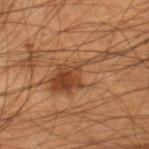| feature | finding |
|---|---|
| notes | catalogued during a skin exam; not biopsied |
| tile lighting | cross-polarized illumination |
| image | ~15 mm tile from a whole-body skin photo |
| patient | male, aged 43–47 |
| TBP lesion metrics | a border-irregularity rating of about 10/10, a within-lesion color-variation index near 4/10, and a peripheral color-asymmetry measure near 1; a nevus-likeness score of about 80/100 and a lesion-detection confidence of about 95/100 |
| body site | the right lower leg |